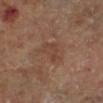follow-up = total-body-photography surveillance lesion; no biopsy
subject = male, approximately 70 years of age
site = the right lower leg
image source = ~15 mm crop, total-body skin-cancer survey
automated metrics = a mean CIELAB color near L≈41 a*≈18 b*≈27 and roughly 5 lightness units darker than nearby skin
lesion diameter = about 4 mm
tile lighting = cross-polarized